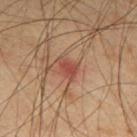patient: male, approximately 65 years of age | lighting: cross-polarized | TBP lesion metrics: a footprint of about 4 mm²; a lesion color around L≈47 a*≈26 b*≈29 in CIELAB and a normalized lesion–skin contrast near 7; a border-irregularity rating of about 3/10, internal color variation of about 1.5 on a 0–10 scale, and a peripheral color-asymmetry measure near 0.5 | site: the mid back | diameter: ≈3 mm | image: ~15 mm crop, total-body skin-cancer survey.